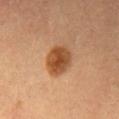image-analysis metrics: a lesion area of about 9.5 mm², an eccentricity of roughly 0.65, and a symmetry-axis asymmetry near 0.15; an average lesion color of about L≈44 a*≈22 b*≈35 (CIELAB), roughly 13 lightness units darker than nearby skin, and a lesion-to-skin contrast of about 10 (normalized; higher = more distinct)
image source: total-body-photography crop, ~15 mm field of view
patient: male, aged 63–67
lesion diameter: ≈4 mm
site: the mid back
lighting: cross-polarized illumination